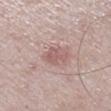Case summary:
– follow-up: imaged on a skin check; not biopsied
– body site: the right lower leg
– lesion diameter: ≈3 mm
– imaging modality: 15 mm crop, total-body photography
– patient: male, aged approximately 60